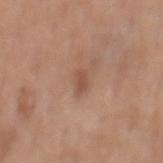Background: Cropped from a total-body skin-imaging series; the visible field is about 15 mm. From the mid back. The patient is a male in their 70s. Captured under white-light illumination. Measured at roughly 2.5 mm in maximum diameter.